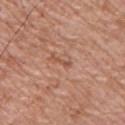image:
  source: total-body photography crop
  field_of_view_mm: 15
patient:
  sex: male
  age_approx: 65
site: chest
lesion_size:
  long_diameter_mm_approx: 2.5
lighting: white-light